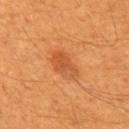This image is a 15 mm lesion crop taken from a total-body photograph.
On the upper back.
A male subject about 60 years old.